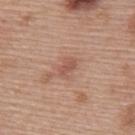Case summary:
• biopsy status · no biopsy performed (imaged during a skin exam)
• body site · the upper back
• diameter · ~2.5 mm (longest diameter)
• acquisition · ~15 mm crop, total-body skin-cancer survey
• tile lighting · white-light
• subject · female, aged approximately 60